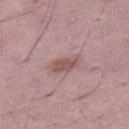acquisition = 15 mm crop, total-body photography; body site = the right thigh; patient = male, aged approximately 55; illumination = white-light illumination.